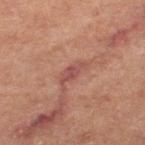  biopsy_status: not biopsied; imaged during a skin examination
  patient:
    sex: female
    age_approx: 60
  automated_metrics:
    cielab_L: 38
    cielab_a: 21
    cielab_b: 20
    vs_skin_darker_L: 7.0
    vs_skin_contrast_norm: 6.5
    border_irregularity_0_10: 3.5
    color_variation_0_10: 1.0
  lesion_size:
    long_diameter_mm_approx: 3.5
  image:
    source: total-body photography crop
    field_of_view_mm: 15
  site: left thigh